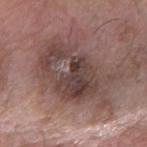Captured during whole-body skin photography for melanoma surveillance; the lesion was not biopsied.
A male subject aged approximately 60.
A close-up tile cropped from a whole-body skin photograph, about 15 mm across.
The lesion is on the right forearm.
Imaged with white-light lighting.
The lesion-visualizer software estimated an average lesion color of about L≈43 a*≈17 b*≈19 (CIELAB) and roughly 11 lightness units darker than nearby skin.
About 9 mm across.